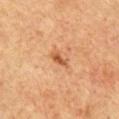Q: Is there a histopathology result?
A: total-body-photography surveillance lesion; no biopsy
Q: What did automated image analysis measure?
A: a mean CIELAB color near L≈50 a*≈24 b*≈36, roughly 11 lightness units darker than nearby skin, and a normalized border contrast of about 7.5; a classifier nevus-likeness of about 20/100 and a detector confidence of about 100 out of 100 that the crop contains a lesion
Q: What kind of image is this?
A: ~15 mm tile from a whole-body skin photo
Q: What are the patient's age and sex?
A: male, about 65 years old
Q: Where on the body is the lesion?
A: the chest
Q: Lesion size?
A: about 2.5 mm
Q: Illumination type?
A: cross-polarized